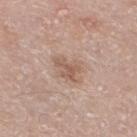Notes:
- notes: total-body-photography surveillance lesion; no biopsy
- location: the leg
- lesion diameter: ≈3.5 mm
- acquisition: total-body-photography crop, ~15 mm field of view
- image-analysis metrics: a lesion color around L≈57 a*≈18 b*≈27 in CIELAB and about 9 CIELAB-L* units darker than the surrounding skin; an automated nevus-likeness rating near 0 out of 100
- patient: male, about 70 years old